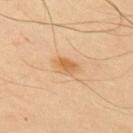{
  "biopsy_status": "not biopsied; imaged during a skin examination",
  "lighting": "cross-polarized",
  "site": "upper back",
  "lesion_size": {
    "long_diameter_mm_approx": 2.5
  },
  "image": {
    "source": "total-body photography crop",
    "field_of_view_mm": 15
  },
  "patient": {
    "sex": "male",
    "age_approx": 50
  }
}